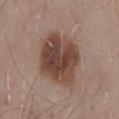Impression:
Recorded during total-body skin imaging; not selected for excision or biopsy.
Context:
The lesion is on the mid back. A male patient about 55 years old. A region of skin cropped from a whole-body photographic capture, roughly 15 mm wide.